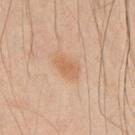A male subject aged 43 to 47. On the right upper arm. Imaged with cross-polarized lighting. A 15 mm crop from a total-body photograph taken for skin-cancer surveillance. The lesion's longest dimension is about 3.5 mm. Automated tile analysis of the lesion measured a shape-asymmetry score of about 0.2 (0 = symmetric). The analysis additionally found an average lesion color of about L≈61 a*≈20 b*≈34 (CIELAB), roughly 7 lightness units darker than nearby skin, and a normalized border contrast of about 6. It also reported a border-irregularity index near 2/10, internal color variation of about 1.5 on a 0–10 scale, and radial color variation of about 0.5. The analysis additionally found a nevus-likeness score of about 60/100 and a detector confidence of about 100 out of 100 that the crop contains a lesion.